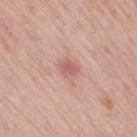<tbp_lesion>
<biopsy_status>not biopsied; imaged during a skin examination</biopsy_status>
<image>
  <source>total-body photography crop</source>
  <field_of_view_mm>15</field_of_view_mm>
</image>
<lighting>white-light</lighting>
<automated_metrics>
  <area_mm2_approx>3.5</area_mm2_approx>
  <shape_asymmetry>0.25</shape_asymmetry>
</automated_metrics>
<patient>
  <sex>female</sex>
  <age_approx>60</age_approx>
</patient>
<site>right thigh</site>
<lesion_size>
  <long_diameter_mm_approx>2.5</long_diameter_mm_approx>
</lesion_size>
</tbp_lesion>